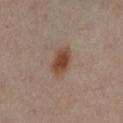Findings:
- notes · no biopsy performed (imaged during a skin exam)
- subject · female, aged around 40
- image · ~15 mm tile from a whole-body skin photo
- location · the right leg